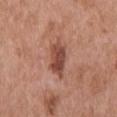Q: Was this lesion biopsied?
A: imaged on a skin check; not biopsied
Q: Who is the patient?
A: male, aged approximately 65
Q: Lesion location?
A: the upper back
Q: What is the imaging modality?
A: ~15 mm tile from a whole-body skin photo